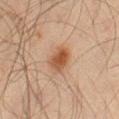Captured during whole-body skin photography for melanoma surveillance; the lesion was not biopsied. This is a cross-polarized tile. Cropped from a total-body skin-imaging series; the visible field is about 15 mm. From the right thigh. The lesion's longest dimension is about 3.5 mm. The patient is a male about 45 years old.